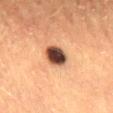Imaged during a routine full-body skin examination; the lesion was not biopsied and no histopathology is available. The tile uses cross-polarized illumination. A region of skin cropped from a whole-body photographic capture, roughly 15 mm wide. Measured at roughly 3.5 mm in maximum diameter. The lesion is on the mid back. The subject is a female aged 53 to 57.